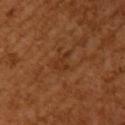Case summary:
• biopsy status — total-body-photography surveillance lesion; no biopsy
• diameter — ~2.5 mm (longest diameter)
• tile lighting — cross-polarized
• automated metrics — a border-irregularity index near 6.5/10 and a within-lesion color-variation index near 0/10
• location — the back
• subject — female, roughly 55 years of age
• imaging modality — ~15 mm tile from a whole-body skin photo